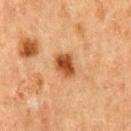follow-up — imaged on a skin check; not biopsied